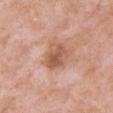biopsy_status: not biopsied; imaged during a skin examination
lesion_size:
  long_diameter_mm_approx: 3.5
site: right upper arm
patient:
  sex: male
  age_approx: 55
image:
  source: total-body photography crop
  field_of_view_mm: 15
lighting: white-light
automated_metrics:
  cielab_L: 56
  cielab_a: 24
  cielab_b: 30
  vs_skin_darker_L: 11.0
  vs_skin_contrast_norm: 7.5
  border_irregularity_0_10: 2.5
  peripheral_color_asymmetry: 1.5
  nevus_likeness_0_100: 0
  lesion_detection_confidence_0_100: 100